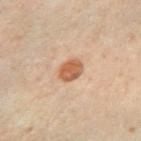Recorded during total-body skin imaging; not selected for excision or biopsy. A 15 mm crop from a total-body photograph taken for skin-cancer surveillance. Approximately 3 mm at its widest. A female subject aged approximately 30. This is a cross-polarized tile. Automated tile analysis of the lesion measured an area of roughly 6 mm² and an outline eccentricity of about 0.4 (0 = round, 1 = elongated). The analysis additionally found a classifier nevus-likeness of about 100/100. The lesion is located on the left arm.